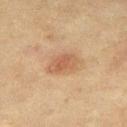Assessment: Part of a total-body skin-imaging series; this lesion was reviewed on a skin check and was not flagged for biopsy. Context: The lesion-visualizer software estimated an area of roughly 6 mm². The analysis additionally found a border-irregularity rating of about 2/10, internal color variation of about 2.5 on a 0–10 scale, and a peripheral color-asymmetry measure near 1. It also reported a classifier nevus-likeness of about 70/100 and a lesion-detection confidence of about 100/100. Approximately 3.5 mm at its widest. A female subject, aged around 55. A 15 mm close-up tile from a total-body photography series done for melanoma screening. On the right thigh. This is a cross-polarized tile.